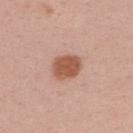The tile uses white-light illumination. The subject is a male aged approximately 40. The lesion is located on the upper back. The lesion-visualizer software estimated a footprint of about 8.5 mm² and an outline eccentricity of about 0.65 (0 = round, 1 = elongated). The software also gave a classifier nevus-likeness of about 100/100 and lesion-presence confidence of about 100/100. A close-up tile cropped from a whole-body skin photograph, about 15 mm across.